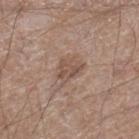biopsy_status: not biopsied; imaged during a skin examination
lighting: white-light
lesion_size:
  long_diameter_mm_approx: 3.0
automated_metrics:
  color_variation_0_10: 2.0
  peripheral_color_asymmetry: 1.0
image:
  source: total-body photography crop
  field_of_view_mm: 15
patient:
  sex: male
  age_approx: 65
site: right lower leg